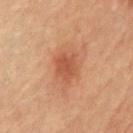biopsy status=imaged on a skin check; not biopsied
TBP lesion metrics=a border-irregularity rating of about 2.5/10, a within-lesion color-variation index near 2.5/10, and a peripheral color-asymmetry measure near 0.5
anatomic site=the chest
imaging modality=15 mm crop, total-body photography
patient=male, aged 83 or older
size=≈3 mm